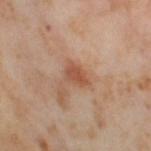body site: the right thigh
size: ≈3 mm
subject: female, roughly 55 years of age
automated lesion analysis: an area of roughly 4.5 mm², a shape eccentricity near 0.75, and two-axis asymmetry of about 0.25; a mean CIELAB color near L≈53 a*≈23 b*≈32, about 9 CIELAB-L* units darker than the surrounding skin, and a normalized border contrast of about 7
image source: ~15 mm tile from a whole-body skin photo